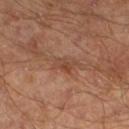Impression:
This lesion was catalogued during total-body skin photography and was not selected for biopsy.
Clinical summary:
Longest diameter approximately 2.5 mm. A 15 mm close-up extracted from a 3D total-body photography capture. The patient is a male about 65 years old. Imaged with cross-polarized lighting. Located on the left lower leg. The lesion-visualizer software estimated a shape eccentricity near 0.8 and a shape-asymmetry score of about 0.4 (0 = symmetric).